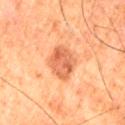{"biopsy_status": "not biopsied; imaged during a skin examination", "site": "left thigh", "patient": {"sex": "male", "age_approx": 80}, "image": {"source": "total-body photography crop", "field_of_view_mm": 15}}